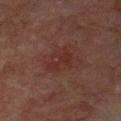biopsy status = catalogued during a skin exam; not biopsied | automated metrics = a lesion color around L≈23 a*≈20 b*≈19 in CIELAB, a lesion–skin lightness drop of about 4, and a normalized border contrast of about 5; a border-irregularity rating of about 5.5/10, a within-lesion color-variation index near 2/10, and peripheral color asymmetry of about 0.5; a lesion-detection confidence of about 100/100 | image = total-body-photography crop, ~15 mm field of view | site = the upper back | subject = male, aged 63 to 67.